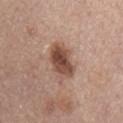The lesion was photographed on a routine skin check and not biopsied; there is no pathology result. Longest diameter approximately 5 mm. From the mid back. This image is a 15 mm lesion crop taken from a total-body photograph. An algorithmic analysis of the crop reported a lesion area of about 12 mm² and a symmetry-axis asymmetry near 0.2. The software also gave a lesion-detection confidence of about 100/100. Imaged with white-light lighting. The subject is a female in their mid- to late 60s.